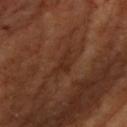The lesion was tiled from a total-body skin photograph and was not biopsied.